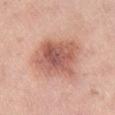biopsy_status: not biopsied; imaged during a skin examination
image:
  source: total-body photography crop
  field_of_view_mm: 15
site: leg
patient:
  sex: female
  age_approx: 55
lighting: white-light
automated_metrics:
  eccentricity: 0.55
  shape_asymmetry: 0.2
  cielab_L: 58
  cielab_a: 25
  cielab_b: 28
  vs_skin_darker_L: 13.0
  border_irregularity_0_10: 2.5
  color_variation_0_10: 6.0
  peripheral_color_asymmetry: 2.0
  nevus_likeness_0_100: 40
  lesion_detection_confidence_0_100: 100